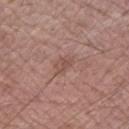workup: no biopsy performed (imaged during a skin exam) | image: ~15 mm crop, total-body skin-cancer survey | tile lighting: white-light | anatomic site: the right upper arm | diameter: ≈2.5 mm | patient: male, in their mid- to late 60s.